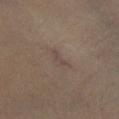Case summary:
- workup: total-body-photography surveillance lesion; no biopsy
- body site: the left lower leg
- subject: female, aged approximately 30
- imaging modality: ~15 mm tile from a whole-body skin photo